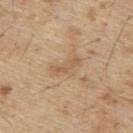Imaged during a routine full-body skin examination; the lesion was not biopsied and no histopathology is available. Captured under white-light illumination. This image is a 15 mm lesion crop taken from a total-body photograph. On the upper back. A male subject, approximately 70 years of age. The recorded lesion diameter is about 3.5 mm.